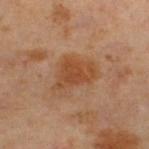Clinical impression:
Part of a total-body skin-imaging series; this lesion was reviewed on a skin check and was not flagged for biopsy.
Image and clinical context:
Located on the leg. The patient is a male in their 60s. The tile uses cross-polarized illumination. A lesion tile, about 15 mm wide, cut from a 3D total-body photograph. Approximately 5 mm at its widest.